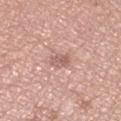Case summary:
• subject: female, aged 48–52
• location: the left lower leg
• illumination: white-light illumination
• size: ≈2.5 mm
• automated lesion analysis: a lesion area of about 4 mm², an outline eccentricity of about 0.75 (0 = round, 1 = elongated), and a symmetry-axis asymmetry near 0.2; an average lesion color of about L≈60 a*≈21 b*≈25 (CIELAB), about 10 CIELAB-L* units darker than the surrounding skin, and a normalized border contrast of about 6; an automated nevus-likeness rating near 0 out of 100 and a lesion-detection confidence of about 100/100
• imaging modality: total-body-photography crop, ~15 mm field of view